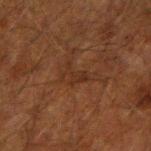workup=imaged on a skin check; not biopsied
lesion size=about 3 mm
illumination=cross-polarized
imaging modality=15 mm crop, total-body photography
anatomic site=the arm
subject=male, in their 50s